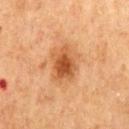Part of a total-body skin-imaging series; this lesion was reviewed on a skin check and was not flagged for biopsy.
Automated image analysis of the tile measured a lesion area of about 13 mm², an eccentricity of roughly 0.65, and a symmetry-axis asymmetry near 0.2. And it measured a border-irregularity index near 2.5/10 and a peripheral color-asymmetry measure near 1.5. The software also gave a classifier nevus-likeness of about 90/100.
This is a cross-polarized tile.
Located on the mid back.
Approximately 4.5 mm at its widest.
A close-up tile cropped from a whole-body skin photograph, about 15 mm across.
A male subject, aged 63 to 67.